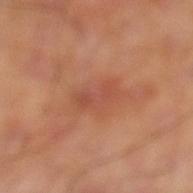Imaged during a routine full-body skin examination; the lesion was not biopsied and no histopathology is available. Located on the left thigh. Cropped from a total-body skin-imaging series; the visible field is about 15 mm. Measured at roughly 4.5 mm in maximum diameter. Automated image analysis of the tile measured a mean CIELAB color near L≈52 a*≈28 b*≈33 and a normalized lesion–skin contrast near 5. The software also gave a border-irregularity rating of about 4/10, a within-lesion color-variation index near 3/10, and radial color variation of about 1. The analysis additionally found lesion-presence confidence of about 100/100.